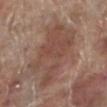From the left lower leg. A male subject approximately 70 years of age. Cropped from a whole-body photographic skin survey; the tile spans about 15 mm. Imaged with white-light lighting.